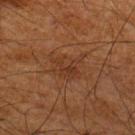The lesion was photographed on a routine skin check and not biopsied; there is no pathology result.
Measured at roughly 4 mm in maximum diameter.
A roughly 15 mm field-of-view crop from a total-body skin photograph.
Located on the upper back.
A male patient, aged 63 to 67.
The lesion-visualizer software estimated a within-lesion color-variation index near 3/10. It also reported a nevus-likeness score of about 5/100.